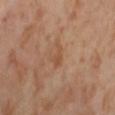Impression:
No biopsy was performed on this lesion — it was imaged during a full skin examination and was not determined to be concerning.
Background:
About 2.5 mm across. The total-body-photography lesion software estimated a shape eccentricity near 0.75 and a shape-asymmetry score of about 0.4 (0 = symmetric). It also reported a peripheral color-asymmetry measure near 0.5. Imaged with cross-polarized lighting. A female patient, about 55 years old. On the right thigh. A lesion tile, about 15 mm wide, cut from a 3D total-body photograph.